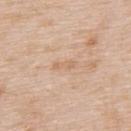Captured during whole-body skin photography for melanoma surveillance; the lesion was not biopsied.
An algorithmic analysis of the crop reported a nevus-likeness score of about 0/100 and lesion-presence confidence of about 100/100.
The patient is a male approximately 65 years of age.
A lesion tile, about 15 mm wide, cut from a 3D total-body photograph.
On the back.